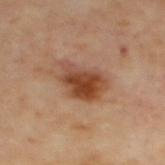Part of a total-body skin-imaging series; this lesion was reviewed on a skin check and was not flagged for biopsy. Captured under cross-polarized illumination. A 15 mm close-up tile from a total-body photography series done for melanoma screening. The lesion-visualizer software estimated roughly 13 lightness units darker than nearby skin and a lesion-to-skin contrast of about 9.5 (normalized; higher = more distinct). From the back.